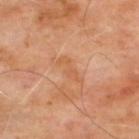Captured during whole-body skin photography for melanoma surveillance; the lesion was not biopsied. Located on the upper back. The recorded lesion diameter is about 4 mm. A roughly 15 mm field-of-view crop from a total-body skin photograph. Captured under cross-polarized illumination. A male subject, aged approximately 65.